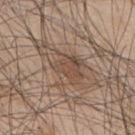– follow-up · no biopsy performed (imaged during a skin exam)